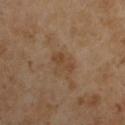• workup: imaged on a skin check; not biopsied
• image-analysis metrics: a mean CIELAB color near L≈43 a*≈17 b*≈32; an automated nevus-likeness rating near 0 out of 100 and lesion-presence confidence of about 100/100
• tile lighting: cross-polarized illumination
• image source: 15 mm crop, total-body photography
• subject: male, aged 53 to 57
• lesion diameter: ≈3 mm
• location: the left upper arm